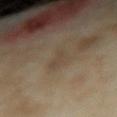A 15 mm close-up tile from a total-body photography series done for melanoma screening. This is a cross-polarized tile. The lesion is on the right forearm. A female subject in their mid-30s. Approximately 3 mm at its widest.